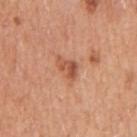Clinical impression: No biopsy was performed on this lesion — it was imaged during a full skin examination and was not determined to be concerning. Acquisition and patient details: On the arm. This image is a 15 mm lesion crop taken from a total-body photograph. A male patient, aged approximately 55.